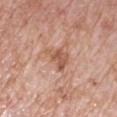Q: Was this lesion biopsied?
A: no biopsy performed (imaged during a skin exam)
Q: Patient demographics?
A: male, in their 70s
Q: How was this image acquired?
A: 15 mm crop, total-body photography
Q: Lesion size?
A: ≈3.5 mm
Q: Illumination type?
A: white-light illumination
Q: What is the anatomic site?
A: the right upper arm